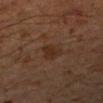site=the left forearm
subject=male, aged approximately 60
imaging modality=~15 mm crop, total-body skin-cancer survey